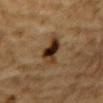Case summary:
• workup — total-body-photography surveillance lesion; no biopsy
• image — ~15 mm tile from a whole-body skin photo
• site — the chest
• size — about 4.5 mm
• subject — male, aged 83 or over
• tile lighting — cross-polarized